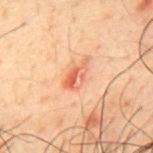biopsy status = no biopsy performed (imaged during a skin exam) | location = the back | lesion diameter = about 3.5 mm | automated metrics = a lesion color around L≈49 a*≈24 b*≈30 in CIELAB and about 9 CIELAB-L* units darker than the surrounding skin; border irregularity of about 3 on a 0–10 scale, a color-variation rating of about 9/10, and peripheral color asymmetry of about 3.5 | acquisition = total-body-photography crop, ~15 mm field of view | subject = male, in their 50s | lighting = cross-polarized.